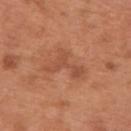workup: catalogued during a skin exam; not biopsied
automated lesion analysis: a footprint of about 7 mm² and a shape eccentricity near 0.9; a border-irregularity rating of about 9.5/10, internal color variation of about 1.5 on a 0–10 scale, and a peripheral color-asymmetry measure near 0
image: ~15 mm tile from a whole-body skin photo
patient: male, aged 63–67
illumination: white-light
body site: the upper back